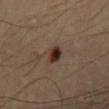Clinical impression: Part of a total-body skin-imaging series; this lesion was reviewed on a skin check and was not flagged for biopsy. Background: A male subject aged approximately 65. Imaged with cross-polarized lighting. Located on the back. Cropped from a whole-body photographic skin survey; the tile spans about 15 mm.